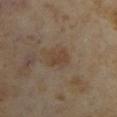  biopsy_status: not biopsied; imaged during a skin examination
  lesion_size:
    long_diameter_mm_approx: 2.5
  patient:
    sex: female
    age_approx: 35
  site: right forearm
  image:
    source: total-body photography crop
    field_of_view_mm: 15
  lighting: cross-polarized